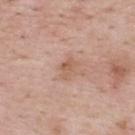The lesion was tiled from a total-body skin photograph and was not biopsied. Imaged with white-light lighting. Automated image analysis of the tile measured an area of roughly 3 mm² and two-axis asymmetry of about 0.4. The software also gave an average lesion color of about L≈58 a*≈21 b*≈30 (CIELAB), about 8 CIELAB-L* units darker than the surrounding skin, and a lesion-to-skin contrast of about 6 (normalized; higher = more distinct). A 15 mm close-up tile from a total-body photography series done for melanoma screening. Longest diameter approximately 2.5 mm. On the back. The subject is a male in their mid-50s.